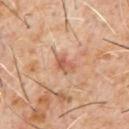The lesion is located on the chest.
A 15 mm crop from a total-body photograph taken for skin-cancer surveillance.
Measured at roughly 3 mm in maximum diameter.
A male subject, aged around 60.
Captured under cross-polarized illumination.